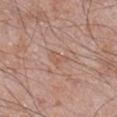Impression:
Recorded during total-body skin imaging; not selected for excision or biopsy.
Background:
Measured at roughly 2.5 mm in maximum diameter. Captured under white-light illumination. On the leg. The patient is a male aged 58 to 62. A region of skin cropped from a whole-body photographic capture, roughly 15 mm wide.